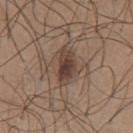notes — no biopsy performed (imaged during a skin exam) | lesion size — ~4.5 mm (longest diameter) | anatomic site — the chest | TBP lesion metrics — a footprint of about 8 mm², an outline eccentricity of about 0.85 (0 = round, 1 = elongated), and two-axis asymmetry of about 0.25; a border-irregularity rating of about 3/10 and a color-variation rating of about 5.5/10; a classifier nevus-likeness of about 85/100 and a lesion-detection confidence of about 100/100 | subject — male, aged around 25 | image — ~15 mm tile from a whole-body skin photo | lighting — white-light.